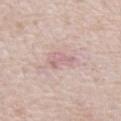On the chest. The subject is a male aged around 80. Cropped from a total-body skin-imaging series; the visible field is about 15 mm.